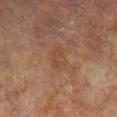Assessment: The lesion was photographed on a routine skin check and not biopsied; there is no pathology result.